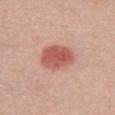notes: catalogued during a skin exam; not biopsied | location: the chest | imaging modality: 15 mm crop, total-body photography | size: about 4.5 mm | illumination: white-light illumination | subject: female, aged around 60.